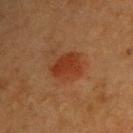{"biopsy_status": "not biopsied; imaged during a skin examination", "image": {"source": "total-body photography crop", "field_of_view_mm": 15}, "patient": {"sex": "female", "age_approx": 40}, "lesion_size": {"long_diameter_mm_approx": 4.0}, "site": "upper back", "lighting": "cross-polarized", "automated_metrics": {"cielab_L": 32, "cielab_a": 24, "cielab_b": 31, "vs_skin_darker_L": 7.0, "vs_skin_contrast_norm": 7.5, "border_irregularity_0_10": 2.5, "nevus_likeness_0_100": 100, "lesion_detection_confidence_0_100": 100}}